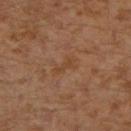This lesion was catalogued during total-body skin photography and was not selected for biopsy.
A lesion tile, about 15 mm wide, cut from a 3D total-body photograph.
The subject is a male approximately 30 years of age.
The lesion is on the left leg.
Longest diameter approximately 3 mm.
This is a cross-polarized tile.